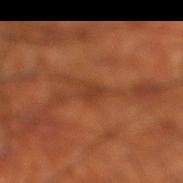workup = imaged on a skin check; not biopsied | lesion size = ~2.5 mm (longest diameter) | automated metrics = a footprint of about 3 mm², an eccentricity of roughly 0.85, and a symmetry-axis asymmetry near 0.4; border irregularity of about 4 on a 0–10 scale, a color-variation rating of about 1/10, and radial color variation of about 0.5; lesion-presence confidence of about 75/100 | tile lighting = cross-polarized | image = ~15 mm crop, total-body skin-cancer survey | patient = male, aged approximately 70 | location = the right thigh.